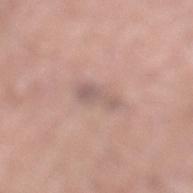Case summary:
- follow-up — catalogued during a skin exam; not biopsied
- patient — male, roughly 45 years of age
- image — ~15 mm tile from a whole-body skin photo
- illumination — white-light illumination
- location — the right lower leg